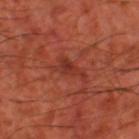Acquisition and patient details:
A 15 mm crop from a total-body photograph taken for skin-cancer surveillance. A subject about 65 years old. The lesion's longest dimension is about 4 mm. From the leg. Captured under cross-polarized illumination.